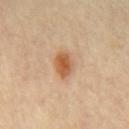biopsy_status: not biopsied; imaged during a skin examination
lesion_size:
  long_diameter_mm_approx: 3.5
lighting: cross-polarized
patient:
  sex: male
  age_approx: 60
image:
  source: total-body photography crop
  field_of_view_mm: 15
site: mid back